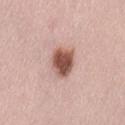Assessment:
Recorded during total-body skin imaging; not selected for excision or biopsy.
Image and clinical context:
A female patient approximately 60 years of age. A 15 mm crop from a total-body photograph taken for skin-cancer surveillance. The lesion's longest dimension is about 4 mm. On the lower back. The tile uses white-light illumination.